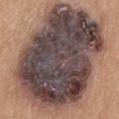Findings:
* anatomic site: the back
* illumination: white-light illumination
* automated metrics: border irregularity of about 2 on a 0–10 scale; a lesion-detection confidence of about 95/100
* subject: female, about 45 years old
* acquisition: 15 mm crop, total-body photography
* size: ≈14.5 mm
* diagnosis: a nodular basal cell carcinoma (malignant)